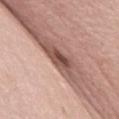Case summary:
* follow-up — imaged on a skin check; not biopsied
* subject — female, about 65 years old
* diameter — ~3 mm (longest diameter)
* anatomic site — the chest
* lighting — white-light illumination
* image — ~15 mm crop, total-body skin-cancer survey
* automated metrics — a mean CIELAB color near L≈47 a*≈22 b*≈25 and a normalized border contrast of about 11.5